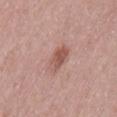Part of a total-body skin-imaging series; this lesion was reviewed on a skin check and was not flagged for biopsy. The recorded lesion diameter is about 3.5 mm. A female patient, aged approximately 40. A 15 mm crop from a total-body photograph taken for skin-cancer surveillance. The lesion is on the back. Captured under white-light illumination.